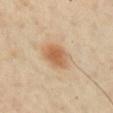| field | value |
|---|---|
| workup | total-body-photography surveillance lesion; no biopsy |
| subject | male, about 65 years old |
| image | total-body-photography crop, ~15 mm field of view |
| automated metrics | an area of roughly 8 mm² and an eccentricity of roughly 0.7; a classifier nevus-likeness of about 100/100 and lesion-presence confidence of about 100/100 |
| anatomic site | the right upper arm |
| illumination | cross-polarized illumination |